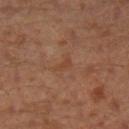No biopsy was performed on this lesion — it was imaged during a full skin examination and was not determined to be concerning. The lesion-visualizer software estimated a mean CIELAB color near L≈42 a*≈21 b*≈30. The software also gave a within-lesion color-variation index near 0/10 and peripheral color asymmetry of about 0. The analysis additionally found a classifier nevus-likeness of about 0/100 and a detector confidence of about 100 out of 100 that the crop contains a lesion. Approximately 2.5 mm at its widest. A male patient aged around 30. Located on the left lower leg. Cropped from a total-body skin-imaging series; the visible field is about 15 mm.